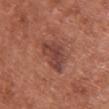{
  "biopsy_status": "not biopsied; imaged during a skin examination",
  "site": "chest",
  "automated_metrics": {
    "area_mm2_approx": 11.0,
    "eccentricity": 0.85,
    "vs_skin_darker_L": 9.0,
    "vs_skin_contrast_norm": 7.5,
    "lesion_detection_confidence_0_100": 100
  },
  "image": {
    "source": "total-body photography crop",
    "field_of_view_mm": 15
  },
  "lesion_size": {
    "long_diameter_mm_approx": 5.5
  },
  "lighting": "white-light",
  "patient": {
    "sex": "female",
    "age_approx": 65
  }
}